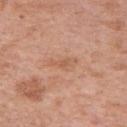Longest diameter approximately 3.5 mm. An algorithmic analysis of the crop reported a shape eccentricity near 0.9 and a shape-asymmetry score of about 0.4 (0 = symmetric). And it measured a border-irregularity index near 4.5/10, internal color variation of about 2 on a 0–10 scale, and a peripheral color-asymmetry measure near 0.5. The analysis additionally found an automated nevus-likeness rating near 0 out of 100 and a lesion-detection confidence of about 100/100. Captured under white-light illumination. A 15 mm close-up extracted from a 3D total-body photography capture. The patient is a female aged around 70. Located on the right upper arm.